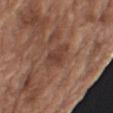Part of a total-body skin-imaging series; this lesion was reviewed on a skin check and was not flagged for biopsy. Imaged with white-light lighting. The patient is a male aged 73–77. The lesion is on the right upper arm. Automated image analysis of the tile measured an eccentricity of roughly 0.6 and a symmetry-axis asymmetry near 0.3. It also reported a lesion-to-skin contrast of about 7 (normalized; higher = more distinct). A 15 mm crop from a total-body photograph taken for skin-cancer surveillance.